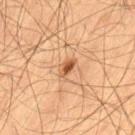Clinical impression: The lesion was tiled from a total-body skin photograph and was not biopsied. Clinical summary: Imaged with cross-polarized lighting. From the left thigh. A male patient, roughly 65 years of age. Longest diameter approximately 2.5 mm. A region of skin cropped from a whole-body photographic capture, roughly 15 mm wide.